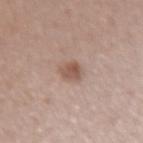No biopsy was performed on this lesion — it was imaged during a full skin examination and was not determined to be concerning.
A female subject, aged 33 to 37.
Imaged with white-light lighting.
Located on the right forearm.
A close-up tile cropped from a whole-body skin photograph, about 15 mm across.
The lesion's longest dimension is about 2.5 mm.